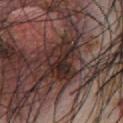Impression: Imaged during a routine full-body skin examination; the lesion was not biopsied and no histopathology is available. Clinical summary: A male subject, aged 53 to 57. The tile uses white-light illumination. This image is a 15 mm lesion crop taken from a total-body photograph. The lesion is located on the chest.